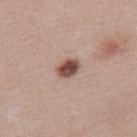biopsy status: no biopsy performed (imaged during a skin exam) | image: ~15 mm tile from a whole-body skin photo | size: ~3 mm (longest diameter) | automated metrics: border irregularity of about 1.5 on a 0–10 scale, internal color variation of about 4.5 on a 0–10 scale, and a peripheral color-asymmetry measure near 1.5; a classifier nevus-likeness of about 100/100 and lesion-presence confidence of about 100/100 | illumination: white-light illumination | patient: male, aged approximately 40 | site: the upper back.